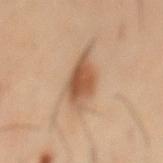workup: catalogued during a skin exam; not biopsied | subject: male, approximately 40 years of age | site: the mid back | automated lesion analysis: an area of roughly 11 mm² and an outline eccentricity of about 0.85 (0 = round, 1 = elongated); roughly 13 lightness units darker than nearby skin and a lesion-to-skin contrast of about 9 (normalized; higher = more distinct); a border-irregularity index near 3/10, internal color variation of about 4.5 on a 0–10 scale, and peripheral color asymmetry of about 1.5; a nevus-likeness score of about 90/100 and a detector confidence of about 100 out of 100 that the crop contains a lesion | acquisition: ~15 mm tile from a whole-body skin photo | lesion diameter: about 6 mm | illumination: cross-polarized illumination.